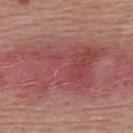notes = total-body-photography surveillance lesion; no biopsy
lighting = white-light
TBP lesion metrics = a lesion area of about 33 mm² and a shape eccentricity near 0.75; roughly 9 lightness units darker than nearby skin and a normalized lesion–skin contrast near 6.5; a color-variation rating of about 5/10 and a peripheral color-asymmetry measure near 1.5
image = total-body-photography crop, ~15 mm field of view
anatomic site = the upper back
diameter = ~10 mm (longest diameter)
subject = female, aged approximately 55